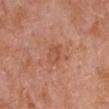No biopsy was performed on this lesion — it was imaged during a full skin examination and was not determined to be concerning. The total-body-photography lesion software estimated an area of roughly 4.5 mm², an eccentricity of roughly 0.7, and a symmetry-axis asymmetry near 0.25. It also reported a lesion–skin lightness drop of about 6 and a normalized lesion–skin contrast near 5. The software also gave a nevus-likeness score of about 0/100 and lesion-presence confidence of about 100/100. The recorded lesion diameter is about 3 mm. A male subject in their mid-60s. A 15 mm close-up extracted from a 3D total-body photography capture. The lesion is on the arm. Captured under white-light illumination.